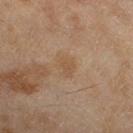Assessment: Recorded during total-body skin imaging; not selected for excision or biopsy.